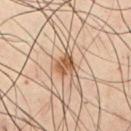Q: Was a biopsy performed?
A: total-body-photography surveillance lesion; no biopsy
Q: How was the tile lit?
A: cross-polarized
Q: What is the anatomic site?
A: the front of the torso
Q: What kind of image is this?
A: ~15 mm crop, total-body skin-cancer survey
Q: Lesion size?
A: ≈2.5 mm
Q: Patient demographics?
A: male, roughly 50 years of age